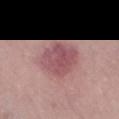Part of a total-body skin-imaging series; this lesion was reviewed on a skin check and was not flagged for biopsy. The recorded lesion diameter is about 4.5 mm. The subject is a male in their 40s. The total-body-photography lesion software estimated a lesion area of about 14 mm², an outline eccentricity of about 0.5 (0 = round, 1 = elongated), and a symmetry-axis asymmetry near 0.15. And it measured a mean CIELAB color near L≈52 a*≈26 b*≈18 and a normalized lesion–skin contrast near 7.5. The lesion is located on the leg. A roughly 15 mm field-of-view crop from a total-body skin photograph.